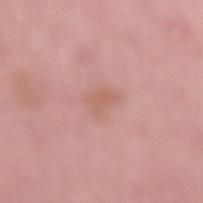<case>
  <biopsy_status>not biopsied; imaged during a skin examination</biopsy_status>
  <lighting>white-light</lighting>
  <lesion_size>
    <long_diameter_mm_approx>2.5</long_diameter_mm_approx>
  </lesion_size>
  <site>back</site>
  <image>
    <source>total-body photography crop</source>
    <field_of_view_mm>15</field_of_view_mm>
  </image>
  <patient>
    <sex>male</sex>
    <age_approx>55</age_approx>
  </patient>
</case>